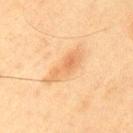follow-up = imaged on a skin check; not biopsied
subject = male, about 45 years old
lesion diameter = ~6 mm (longest diameter)
image source = ~15 mm crop, total-body skin-cancer survey
illumination = cross-polarized
site = the upper back